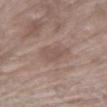Q: Was this lesion biopsied?
A: total-body-photography surveillance lesion; no biopsy
Q: Lesion size?
A: ≈3.5 mm
Q: What is the imaging modality?
A: ~15 mm crop, total-body skin-cancer survey
Q: Lesion location?
A: the right upper arm
Q: Patient demographics?
A: female, approximately 70 years of age
Q: What lighting was used for the tile?
A: white-light
Q: Automated lesion metrics?
A: an automated nevus-likeness rating near 0 out of 100 and lesion-presence confidence of about 100/100